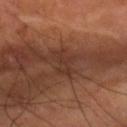Context:
Cropped from a total-body skin-imaging series; the visible field is about 15 mm. The lesion's longest dimension is about 3.5 mm. A male subject, in their mid-50s. Captured under cross-polarized illumination. The lesion-visualizer software estimated a footprint of about 5.5 mm² and an eccentricity of roughly 0.85. The analysis additionally found a border-irregularity index near 4.5/10 and a within-lesion color-variation index near 1/10. The lesion is located on the right forearm.